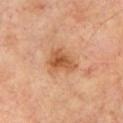Q: Was a biopsy performed?
A: imaged on a skin check; not biopsied
Q: Illumination type?
A: cross-polarized illumination
Q: How large is the lesion?
A: ≈4 mm
Q: What did automated image analysis measure?
A: an average lesion color of about L≈55 a*≈24 b*≈37 (CIELAB), roughly 11 lightness units darker than nearby skin, and a normalized lesion–skin contrast near 8; an automated nevus-likeness rating near 25 out of 100 and a detector confidence of about 100 out of 100 that the crop contains a lesion
Q: How was this image acquired?
A: ~15 mm tile from a whole-body skin photo
Q: What are the patient's age and sex?
A: male, aged around 70
Q: Lesion location?
A: the chest